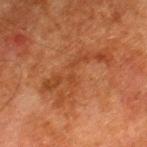Captured during whole-body skin photography for melanoma surveillance; the lesion was not biopsied.
A male subject aged 78 to 82.
Approximately 8.5 mm at its widest.
From the left thigh.
A 15 mm crop from a total-body photograph taken for skin-cancer surveillance.